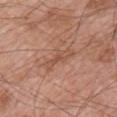Assessment: Captured during whole-body skin photography for melanoma surveillance; the lesion was not biopsied. Clinical summary: Located on the chest. Captured under white-light illumination. Approximately 4.5 mm at its widest. A male patient about 70 years old. Cropped from a whole-body photographic skin survey; the tile spans about 15 mm.